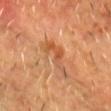Clinical summary:
The lesion's longest dimension is about 5.5 mm. Captured under cross-polarized illumination. From the chest. A 15 mm close-up extracted from a 3D total-body photography capture. A male subject, aged approximately 60. Automated tile analysis of the lesion measured a footprint of about 7 mm² and two-axis asymmetry of about 0.55. And it measured a mean CIELAB color near L≈50 a*≈24 b*≈37, a lesion–skin lightness drop of about 8, and a normalized border contrast of about 6.